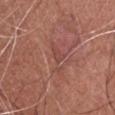The lesion was photographed on a routine skin check and not biopsied; there is no pathology result. The total-body-photography lesion software estimated a footprint of about 1.5 mm², an outline eccentricity of about 0.75 (0 = round, 1 = elongated), and a shape-asymmetry score of about 0.2 (0 = symmetric). The software also gave border irregularity of about 1.5 on a 0–10 scale, internal color variation of about 0 on a 0–10 scale, and a peripheral color-asymmetry measure near 0. Imaged with white-light lighting. Approximately 1.5 mm at its widest. The patient is a male aged 43 to 47. This image is a 15 mm lesion crop taken from a total-body photograph. From the head or neck.